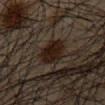Assessment: Recorded during total-body skin imaging; not selected for excision or biopsy. Image and clinical context: The patient is a male roughly 65 years of age. Cropped from a whole-body photographic skin survey; the tile spans about 15 mm. The recorded lesion diameter is about 4 mm. The lesion is located on the chest. This is a cross-polarized tile.